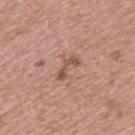Background:
About 3.5 mm across. The tile uses white-light illumination. Located on the upper back. A female patient, aged around 40. A region of skin cropped from a whole-body photographic capture, roughly 15 mm wide.